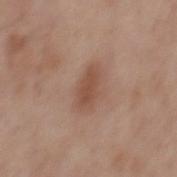| key | value |
|---|---|
| biopsy status | catalogued during a skin exam; not biopsied |
| image source | ~15 mm crop, total-body skin-cancer survey |
| location | the mid back |
| diameter | about 4 mm |
| patient | male, aged 53–57 |